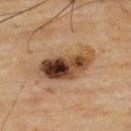Background: Automated tile analysis of the lesion measured a border-irregularity rating of about 3/10, a within-lesion color-variation index near 10/10, and radial color variation of about 7. The lesion is on the upper back. About 7 mm across. A male patient, aged approximately 65. The tile uses cross-polarized illumination. A 15 mm close-up extracted from a 3D total-body photography capture.